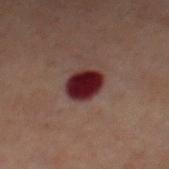| feature | finding |
|---|---|
| patient | male, aged around 60 |
| lighting | cross-polarized |
| body site | the chest |
| lesion diameter | ~3.5 mm (longest diameter) |
| automated metrics | a symmetry-axis asymmetry near 0.1; an average lesion color of about L≈18 a*≈22 b*≈14 (CIELAB) and a normalized border contrast of about 17.5; a within-lesion color-variation index near 3/10 and peripheral color asymmetry of about 1 |
| acquisition | total-body-photography crop, ~15 mm field of view |